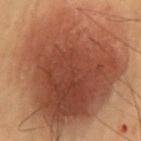Imaged during a routine full-body skin examination; the lesion was not biopsied and no histopathology is available. About 15 mm across. Captured under cross-polarized illumination. The lesion is located on the lower back. This image is a 15 mm lesion crop taken from a total-body photograph. Automated image analysis of the tile measured a footprint of about 125 mm², a shape eccentricity near 0.55, and a shape-asymmetry score of about 0.2 (0 = symmetric). And it measured an average lesion color of about L≈38 a*≈20 b*≈27 (CIELAB), about 12 CIELAB-L* units darker than the surrounding skin, and a lesion-to-skin contrast of about 10.5 (normalized; higher = more distinct). It also reported a classifier nevus-likeness of about 100/100 and lesion-presence confidence of about 100/100. A male patient, about 55 years old.